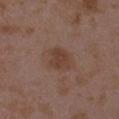Clinical impression:
Imaged during a routine full-body skin examination; the lesion was not biopsied and no histopathology is available.
Background:
A roughly 15 mm field-of-view crop from a total-body skin photograph. The tile uses white-light illumination. The subject is a female aged around 35. The recorded lesion diameter is about 3 mm. From the left upper arm.